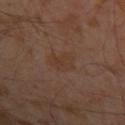* biopsy status: catalogued during a skin exam; not biopsied
* tile lighting: cross-polarized
* acquisition: ~15 mm crop, total-body skin-cancer survey
* automated metrics: internal color variation of about 2.5 on a 0–10 scale and peripheral color asymmetry of about 1; an automated nevus-likeness rating near 0 out of 100 and lesion-presence confidence of about 100/100
* diameter: about 4 mm
* patient: male, aged 28 to 32
* body site: the arm